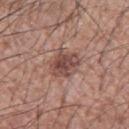workup — total-body-photography surveillance lesion; no biopsy | size — about 4 mm | TBP lesion metrics — a lesion area of about 9.5 mm², an eccentricity of roughly 0.55, and a shape-asymmetry score of about 0.2 (0 = symmetric); an average lesion color of about L≈47 a*≈20 b*≈23 (CIELAB) and a normalized lesion–skin contrast near 8.5 | illumination — white-light illumination | anatomic site — the left forearm | image — total-body-photography crop, ~15 mm field of view | subject — male, approximately 55 years of age.